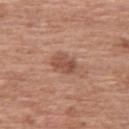Recorded during total-body skin imaging; not selected for excision or biopsy. The subject is a male in their mid-60s. A close-up tile cropped from a whole-body skin photograph, about 15 mm across. The lesion is located on the left upper arm.